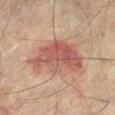Clinical impression: Part of a total-body skin-imaging series; this lesion was reviewed on a skin check and was not flagged for biopsy. Background: A male patient, aged around 75. Located on the right lower leg. Imaged with cross-polarized lighting. Cropped from a whole-body photographic skin survey; the tile spans about 15 mm. Automated tile analysis of the lesion measured a footprint of about 21 mm², a shape eccentricity near 0.75, and a shape-asymmetry score of about 0.35 (0 = symmetric). It also reported an average lesion color of about L≈46 a*≈21 b*≈23 (CIELAB) and a normalized lesion–skin contrast near 7.5. And it measured a classifier nevus-likeness of about 90/100. The recorded lesion diameter is about 7 mm.